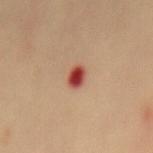workup: no biopsy performed (imaged during a skin exam)
lesion diameter: ~2.5 mm (longest diameter)
subject: female, approximately 50 years of age
lighting: cross-polarized
image source: ~15 mm crop, total-body skin-cancer survey
body site: the back
automated lesion analysis: a mean CIELAB color near L≈47 a*≈32 b*≈30, a lesion–skin lightness drop of about 17, and a normalized border contrast of about 11.5; a classifier nevus-likeness of about 0/100 and lesion-presence confidence of about 100/100